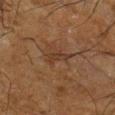Notes:
– body site: the right lower leg
– automated metrics: a footprint of about 3.5 mm², a shape eccentricity near 0.85, and a shape-asymmetry score of about 0.4 (0 = symmetric); an automated nevus-likeness rating near 0 out of 100 and a lesion-detection confidence of about 70/100
– tile lighting: cross-polarized illumination
– lesion size: about 3 mm
– acquisition: ~15 mm crop, total-body skin-cancer survey
– patient: male, in their mid-60s